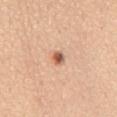workup: total-body-photography surveillance lesion; no biopsy
lesion size: about 2 mm
tile lighting: white-light
subject: female, roughly 45 years of age
automated lesion analysis: an average lesion color of about L≈59 a*≈24 b*≈32 (CIELAB)
acquisition: ~15 mm tile from a whole-body skin photo
anatomic site: the chest